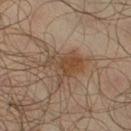workup=imaged on a skin check; not biopsied | image source=total-body-photography crop, ~15 mm field of view | patient=male, about 65 years old | image-analysis metrics=roughly 8 lightness units darker than nearby skin and a normalized lesion–skin contrast near 7; a within-lesion color-variation index near 4.5/10 and radial color variation of about 1.5 | tile lighting=cross-polarized illumination | anatomic site=the right lower leg | lesion diameter=≈4.5 mm.